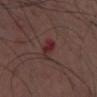Background: The tile uses white-light illumination. From the chest. A 15 mm close-up extracted from a 3D total-body photography capture. A male patient, aged 28 to 32.